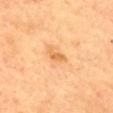This lesion was catalogued during total-body skin photography and was not selected for biopsy. The patient is a female aged 58 to 62. On the upper back. This image is a 15 mm lesion crop taken from a total-body photograph. Imaged with cross-polarized lighting.